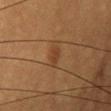biopsy status = imaged on a skin check; not biopsied | subject = female, about 55 years old | site = the head or neck | tile lighting = cross-polarized | size = ~2.5 mm (longest diameter) | image = ~15 mm crop, total-body skin-cancer survey.